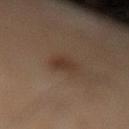Findings:
- follow-up: total-body-photography surveillance lesion; no biopsy
- patient: male, aged 58 to 62
- body site: the right lower leg
- image: ~15 mm tile from a whole-body skin photo